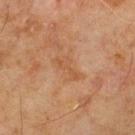Q: Was a biopsy performed?
A: no biopsy performed (imaged during a skin exam)
Q: How was the tile lit?
A: cross-polarized
Q: What is the anatomic site?
A: the upper back
Q: Patient demographics?
A: male, in their 70s
Q: How was this image acquired?
A: total-body-photography crop, ~15 mm field of view
Q: What is the lesion's diameter?
A: about 4 mm